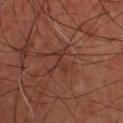Notes:
• biopsy status — imaged on a skin check; not biopsied
• subject — male, aged 58 to 62
• size — about 2.5 mm
• lighting — cross-polarized
• image — 15 mm crop, total-body photography
• body site — the front of the torso